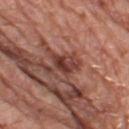Findings:
* follow-up — imaged on a skin check; not biopsied
* acquisition — ~15 mm tile from a whole-body skin photo
* subject — male, in their 80s
* body site — the leg